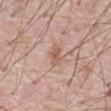The lesion was tiled from a total-body skin photograph and was not biopsied.